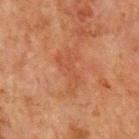Findings:
– follow-up — total-body-photography surveillance lesion; no biopsy
– tile lighting — cross-polarized illumination
– lesion diameter — about 5 mm
– TBP lesion metrics — a shape eccentricity near 0.9 and a symmetry-axis asymmetry near 0.5; a border-irregularity rating of about 7/10 and peripheral color asymmetry of about 0.5; a nevus-likeness score of about 0/100 and a lesion-detection confidence of about 100/100
– location — the chest
– subject — male, aged 63 to 67
– image — ~15 mm crop, total-body skin-cancer survey